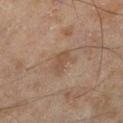  biopsy_status: not biopsied; imaged during a skin examination
  site: left lower leg
  image:
    source: total-body photography crop
    field_of_view_mm: 15
  automated_metrics:
    area_mm2_approx: 4.0
    eccentricity: 0.8
    shape_asymmetry: 0.3
    nevus_likeness_0_100: 0
  patient:
    sex: male
    age_approx: 45
  lighting: cross-polarized